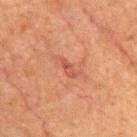workup: no biopsy performed (imaged during a skin exam) | anatomic site: the abdomen | lesion diameter: ≈3.5 mm | image-analysis metrics: a mean CIELAB color near L≈42 a*≈25 b*≈26; a nevus-likeness score of about 0/100 and lesion-presence confidence of about 95/100 | patient: male, roughly 80 years of age | acquisition: 15 mm crop, total-body photography.